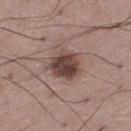<case>
  <biopsy_status>not biopsied; imaged during a skin examination</biopsy_status>
  <lighting>white-light</lighting>
  <site>left thigh</site>
  <lesion_size>
    <long_diameter_mm_approx>4.0</long_diameter_mm_approx>
  </lesion_size>
  <image>
    <source>total-body photography crop</source>
    <field_of_view_mm>15</field_of_view_mm>
  </image>
  <patient>
    <sex>male</sex>
    <age_approx>50</age_approx>
  </patient>
</case>